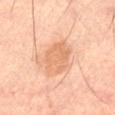Q: What is the lesion's diameter?
A: ≈4.5 mm
Q: Patient demographics?
A: male, approximately 60 years of age
Q: Lesion location?
A: the left thigh
Q: What did automated image analysis measure?
A: an area of roughly 11 mm², an outline eccentricity of about 0.7 (0 = round, 1 = elongated), and a symmetry-axis asymmetry near 0.15; a lesion color around L≈65 a*≈22 b*≈35 in CIELAB, about 8 CIELAB-L* units darker than the surrounding skin, and a normalized border contrast of about 5.5; a color-variation rating of about 2.5/10 and a peripheral color-asymmetry measure near 1
Q: What is the imaging modality?
A: ~15 mm tile from a whole-body skin photo
Q: Illumination type?
A: cross-polarized